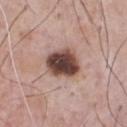The lesion was tiled from a total-body skin photograph and was not biopsied. Imaged with white-light lighting. This image is a 15 mm lesion crop taken from a total-body photograph. The subject is a male approximately 55 years of age. Approximately 4 mm at its widest.